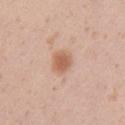Q: Is there a histopathology result?
A: imaged on a skin check; not biopsied
Q: What is the imaging modality?
A: total-body-photography crop, ~15 mm field of view
Q: Where on the body is the lesion?
A: the left upper arm
Q: How large is the lesion?
A: ~2.5 mm (longest diameter)
Q: Illumination type?
A: white-light illumination
Q: What are the patient's age and sex?
A: female, aged 18 to 22